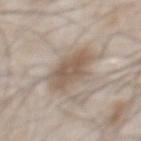Part of a total-body skin-imaging series; this lesion was reviewed on a skin check and was not flagged for biopsy.
A male patient aged around 55.
A lesion tile, about 15 mm wide, cut from a 3D total-body photograph.
On the chest.
The tile uses white-light illumination.
The lesion-visualizer software estimated an area of roughly 13 mm², an outline eccentricity of about 0.65 (0 = round, 1 = elongated), and a symmetry-axis asymmetry near 0.2.
The recorded lesion diameter is about 4.5 mm.